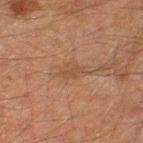size = ~2.5 mm (longest diameter) | image = ~15 mm crop, total-body skin-cancer survey | site = the left thigh | TBP lesion metrics = a lesion area of about 3.5 mm², a shape eccentricity near 0.8, and two-axis asymmetry of about 0.35; a border-irregularity index near 3.5/10 and internal color variation of about 0.5 on a 0–10 scale; a nevus-likeness score of about 0/100 | subject = male, aged around 60 | tile lighting = cross-polarized illumination.